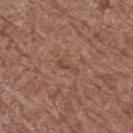{"biopsy_status": "not biopsied; imaged during a skin examination", "patient": {"sex": "female", "age_approx": 75}, "image": {"source": "total-body photography crop", "field_of_view_mm": 15}, "site": "right thigh"}